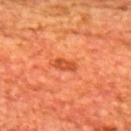patient: male, in their mid- to late 60s | imaging modality: ~15 mm tile from a whole-body skin photo | anatomic site: the upper back.